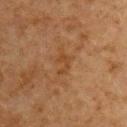Recorded during total-body skin imaging; not selected for excision or biopsy.
The total-body-photography lesion software estimated a classifier nevus-likeness of about 0/100 and a lesion-detection confidence of about 100/100.
Cropped from a total-body skin-imaging series; the visible field is about 15 mm.
Located on the front of the torso.
The patient is a male about 60 years old.
The lesion's longest dimension is about 3 mm.